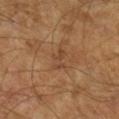  biopsy_status: not biopsied; imaged during a skin examination
  patient:
    sex: male
    age_approx: 65
  image:
    source: total-body photography crop
    field_of_view_mm: 15